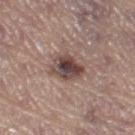notes: total-body-photography surveillance lesion; no biopsy | image: ~15 mm crop, total-body skin-cancer survey | location: the right thigh | subject: female, aged 63–67 | lesion size: ≈4 mm | automated metrics: a lesion area of about 11 mm²; an average lesion color of about L≈46 a*≈16 b*≈20 (CIELAB) and a lesion–skin lightness drop of about 13.